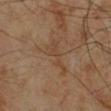Impression: Imaged during a routine full-body skin examination; the lesion was not biopsied and no histopathology is available. Context: The lesion is located on the right lower leg. A 15 mm close-up tile from a total-body photography series done for melanoma screening. Measured at roughly 5 mm in maximum diameter. Captured under cross-polarized illumination. The subject is a male aged approximately 70. The total-body-photography lesion software estimated a lesion area of about 6.5 mm². And it measured a lesion color around L≈42 a*≈17 b*≈30 in CIELAB, a lesion–skin lightness drop of about 5, and a normalized lesion–skin contrast near 5. The analysis additionally found border irregularity of about 8 on a 0–10 scale.